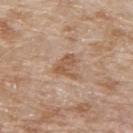This lesion was catalogued during total-body skin photography and was not selected for biopsy.